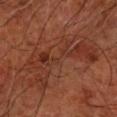Captured during whole-body skin photography for melanoma surveillance; the lesion was not biopsied.
Imaged with cross-polarized lighting.
The lesion is located on the left forearm.
Longest diameter approximately 11.5 mm.
A 15 mm close-up tile from a total-body photography series done for melanoma screening.
The patient is aged 63 to 67.
The total-body-photography lesion software estimated an area of roughly 31 mm², an eccentricity of roughly 0.95, and a symmetry-axis asymmetry near 0.55. The analysis additionally found an average lesion color of about L≈34 a*≈25 b*≈29 (CIELAB) and roughly 6 lightness units darker than nearby skin. It also reported internal color variation of about 6 on a 0–10 scale and peripheral color asymmetry of about 2. And it measured an automated nevus-likeness rating near 0 out of 100 and a lesion-detection confidence of about 80/100.